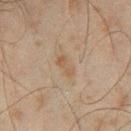{"biopsy_status": "not biopsied; imaged during a skin examination", "image": {"source": "total-body photography crop", "field_of_view_mm": 15}, "patient": {"sex": "male", "age_approx": 45}, "automated_metrics": {"vs_skin_darker_L": 5.0, "vs_skin_contrast_norm": 5.5, "nevus_likeness_0_100": 0, "lesion_detection_confidence_0_100": 100}, "site": "left thigh"}